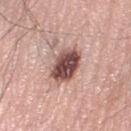The lesion was photographed on a routine skin check and not biopsied; there is no pathology result. A 15 mm crop from a total-body photograph taken for skin-cancer surveillance. Captured under white-light illumination. The lesion is located on the left lower leg. The subject is a male roughly 60 years of age. The total-body-photography lesion software estimated border irregularity of about 2 on a 0–10 scale and internal color variation of about 6.5 on a 0–10 scale. The software also gave a nevus-likeness score of about 60/100.